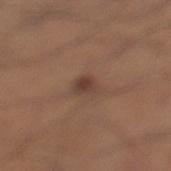Findings:
* notes — no biopsy performed (imaged during a skin exam)
* site — the leg
* subject — male, in their mid-30s
* image — ~15 mm tile from a whole-body skin photo
* diameter — about 2.5 mm
* lighting — white-light illumination